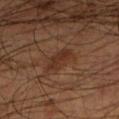| feature | finding |
|---|---|
| notes | catalogued during a skin exam; not biopsied |
| size | ~4 mm (longest diameter) |
| imaging modality | total-body-photography crop, ~15 mm field of view |
| location | the left lower leg |
| tile lighting | cross-polarized |
| patient | male, aged 53 to 57 |